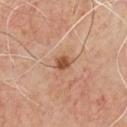Q: Is there a histopathology result?
A: total-body-photography surveillance lesion; no biopsy
Q: Automated lesion metrics?
A: an area of roughly 3 mm² and a shape eccentricity near 0.45; a lesion color around L≈50 a*≈23 b*≈33 in CIELAB, roughly 12 lightness units darker than nearby skin, and a lesion-to-skin contrast of about 9 (normalized; higher = more distinct); a within-lesion color-variation index near 1.5/10 and peripheral color asymmetry of about 0.5; an automated nevus-likeness rating near 90 out of 100
Q: How was this image acquired?
A: total-body-photography crop, ~15 mm field of view
Q: Illumination type?
A: cross-polarized
Q: Lesion location?
A: the chest
Q: Who is the patient?
A: male, aged 48 to 52